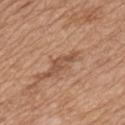<tbp_lesion>
<biopsy_status>not biopsied; imaged during a skin examination</biopsy_status>
<lesion_size>
  <long_diameter_mm_approx>3.5</long_diameter_mm_approx>
</lesion_size>
<automated_metrics>
  <area_mm2_approx>3.5</area_mm2_approx>
  <eccentricity>0.95</eccentricity>
  <shape_asymmetry>0.5</shape_asymmetry>
  <cielab_L>51</cielab_L>
  <cielab_a>21</cielab_a>
  <cielab_b>31</cielab_b>
  <vs_skin_contrast_norm>6.5</vs_skin_contrast_norm>
  <border_irregularity_0_10>6.5</border_irregularity_0_10>
  <peripheral_color_asymmetry>0.0</peripheral_color_asymmetry>
  <nevus_likeness_0_100>0</nevus_likeness_0_100>
</automated_metrics>
<lighting>white-light</lighting>
<image>
  <source>total-body photography crop</source>
  <field_of_view_mm>15</field_of_view_mm>
</image>
<patient>
  <sex>male</sex>
  <age_approx>65</age_approx>
</patient>
<site>chest</site>
</tbp_lesion>